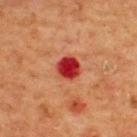The lesion was photographed on a routine skin check and not biopsied; there is no pathology result. Captured under cross-polarized illumination. A male subject, aged 63–67. A roughly 15 mm field-of-view crop from a total-body skin photograph. Located on the back. Longest diameter approximately 2.5 mm.